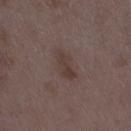Clinical summary:
Measured at roughly 4 mm in maximum diameter. Cropped from a total-body skin-imaging series; the visible field is about 15 mm. The patient is a female aged 33–37. This is a white-light tile. The lesion is located on the leg.